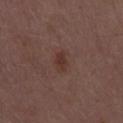Notes:
• biopsy status: imaged on a skin check; not biopsied
• lesion diameter: ≈3 mm
• patient: male, aged 48 to 52
• image-analysis metrics: a footprint of about 3.5 mm², an eccentricity of roughly 0.9, and two-axis asymmetry of about 0.3; an average lesion color of about L≈33 a*≈18 b*≈22 (CIELAB) and a normalized lesion–skin contrast near 7; a border-irregularity index near 3.5/10, a within-lesion color-variation index near 2/10, and peripheral color asymmetry of about 1; a classifier nevus-likeness of about 45/100 and a detector confidence of about 100 out of 100 that the crop contains a lesion
• anatomic site: the mid back
• tile lighting: white-light illumination
• acquisition: total-body-photography crop, ~15 mm field of view